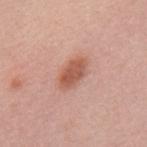The lesion was photographed on a routine skin check and not biopsied; there is no pathology result. Measured at roughly 4 mm in maximum diameter. On the back. A female subject, aged 58–62. A 15 mm crop from a total-body photograph taken for skin-cancer surveillance. Captured under white-light illumination.